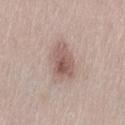{
  "biopsy_status": "not biopsied; imaged during a skin examination",
  "lesion_size": {
    "long_diameter_mm_approx": 4.5
  },
  "image": {
    "source": "total-body photography crop",
    "field_of_view_mm": 15
  },
  "patient": {
    "sex": "female",
    "age_approx": 65
  },
  "automated_metrics": {
    "border_irregularity_0_10": 3.0,
    "color_variation_0_10": 5.5,
    "peripheral_color_asymmetry": 2.0
  },
  "lighting": "white-light",
  "site": "left thigh"
}